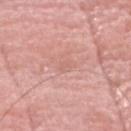No biopsy was performed on this lesion — it was imaged during a full skin examination and was not determined to be concerning.
A region of skin cropped from a whole-body photographic capture, roughly 15 mm wide.
From the head or neck.
A male patient, in their 70s.